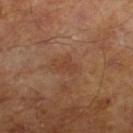Recorded during total-body skin imaging; not selected for excision or biopsy. This is a cross-polarized tile. Cropped from a whole-body photographic skin survey; the tile spans about 15 mm. About 2.5 mm across. The patient is a male approximately 65 years of age. The lesion-visualizer software estimated an eccentricity of roughly 0.85 and two-axis asymmetry of about 0.5. The analysis additionally found an average lesion color of about L≈40 a*≈22 b*≈30 (CIELAB), a lesion–skin lightness drop of about 6, and a lesion-to-skin contrast of about 5 (normalized; higher = more distinct).